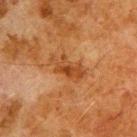A male subject, aged approximately 80. Located on the upper back. The recorded lesion diameter is about 4.5 mm. A close-up tile cropped from a whole-body skin photograph, about 15 mm across.